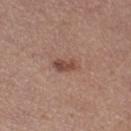This lesion was catalogued during total-body skin photography and was not selected for biopsy.
Located on the right thigh.
Captured under white-light illumination.
The total-body-photography lesion software estimated a footprint of about 4 mm², an eccentricity of roughly 0.85, and a shape-asymmetry score of about 0.25 (0 = symmetric). It also reported a lesion color around L≈47 a*≈21 b*≈25 in CIELAB and a lesion-to-skin contrast of about 8 (normalized; higher = more distinct).
The patient is a female aged 33 to 37.
This image is a 15 mm lesion crop taken from a total-body photograph.
Approximately 3 mm at its widest.